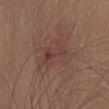The lesion was photographed on a routine skin check and not biopsied; there is no pathology result. A female patient aged 28 to 32. From the head or neck. Cropped from a total-body skin-imaging series; the visible field is about 15 mm.